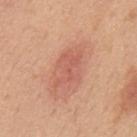Assessment:
Recorded during total-body skin imaging; not selected for excision or biopsy.
Background:
The lesion-visualizer software estimated an average lesion color of about L≈59 a*≈27 b*≈31 (CIELAB) and about 8 CIELAB-L* units darker than the surrounding skin. It also reported a border-irregularity index near 3.5/10, a within-lesion color-variation index near 3/10, and peripheral color asymmetry of about 1. It also reported a detector confidence of about 100 out of 100 that the crop contains a lesion. A male subject approximately 50 years of age. Approximately 5 mm at its widest. This is a white-light tile. A region of skin cropped from a whole-body photographic capture, roughly 15 mm wide. On the back.